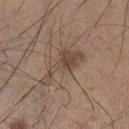notes=total-body-photography surveillance lesion; no biopsy | patient=male, approximately 35 years of age | diameter=about 6 mm | lighting=white-light illumination | image source=15 mm crop, total-body photography | location=the leg.